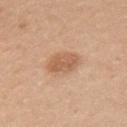| feature | finding |
|---|---|
| biopsy status | imaged on a skin check; not biopsied |
| illumination | white-light illumination |
| patient | female, in their 40s |
| imaging modality | 15 mm crop, total-body photography |
| diameter | ≈4 mm |
| TBP lesion metrics | a border-irregularity rating of about 2/10, internal color variation of about 2.5 on a 0–10 scale, and radial color variation of about 1 |
| site | the left upper arm |